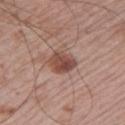Recorded during total-body skin imaging; not selected for excision or biopsy. A lesion tile, about 15 mm wide, cut from a 3D total-body photograph. A male patient, approximately 65 years of age. This is a white-light tile. The lesion's longest dimension is about 3 mm. The lesion is located on the left upper arm.